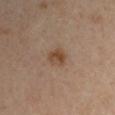The lesion was photographed on a routine skin check and not biopsied; there is no pathology result. The lesion is located on the left upper arm. The subject is a male approximately 45 years of age. Imaged with cross-polarized lighting. A region of skin cropped from a whole-body photographic capture, roughly 15 mm wide. Measured at roughly 2.5 mm in maximum diameter.